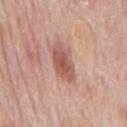biopsy status: total-body-photography surveillance lesion; no biopsy | site: the mid back | acquisition: ~15 mm crop, total-body skin-cancer survey | patient: male, in their mid- to late 70s | automated lesion analysis: a lesion area of about 8.5 mm², an outline eccentricity of about 0.85 (0 = round, 1 = elongated), and a symmetry-axis asymmetry near 0.2.